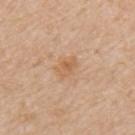biopsy_status: not biopsied; imaged during a skin examination
lesion_size:
  long_diameter_mm_approx: 2.5
image:
  source: total-body photography crop
  field_of_view_mm: 15
automated_metrics:
  area_mm2_approx: 3.5
  eccentricity: 0.75
  cielab_L: 61
  cielab_a: 20
  cielab_b: 36
  vs_skin_contrast_norm: 6.0
  nevus_likeness_0_100: 5
  lesion_detection_confidence_0_100: 100
patient:
  sex: male
  age_approx: 70
lighting: white-light
site: mid back